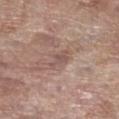Clinical impression:
Recorded during total-body skin imaging; not selected for excision or biopsy.
Acquisition and patient details:
Approximately 2.5 mm at its widest. Located on the right lower leg. Automated tile analysis of the lesion measured a footprint of about 3 mm², a shape eccentricity near 0.8, and a shape-asymmetry score of about 0.4 (0 = symmetric). A 15 mm crop from a total-body photograph taken for skin-cancer surveillance. A female subject in their mid-80s or older.